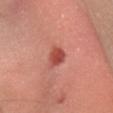  image:
    source: total-body photography crop
    field_of_view_mm: 15
  patient:
    sex: female
    age_approx: 35
  site: head or neck
  lesion_size:
    long_diameter_mm_approx: 3.0